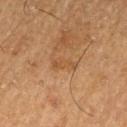Findings:
* biopsy status · catalogued during a skin exam; not biopsied
* automated lesion analysis · a lesion area of about 3.5 mm² and a shape eccentricity near 0.9; a lesion–skin lightness drop of about 5 and a normalized lesion–skin contrast near 5; a color-variation rating of about 1/10 and radial color variation of about 0.5; a nevus-likeness score of about 0/100 and a detector confidence of about 100 out of 100 that the crop contains a lesion
* imaging modality · 15 mm crop, total-body photography
* body site · the left thigh
* patient · male, approximately 75 years of age